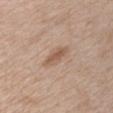Clinical impression: No biopsy was performed on this lesion — it was imaged during a full skin examination and was not determined to be concerning. Image and clinical context: This image is a 15 mm lesion crop taken from a total-body photograph. A female subject, about 40 years old. Approximately 3.5 mm at its widest. The lesion is located on the chest.